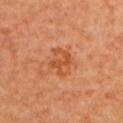Assessment: Part of a total-body skin-imaging series; this lesion was reviewed on a skin check and was not flagged for biopsy. Clinical summary: The subject is female. On the back. Cropped from a total-body skin-imaging series; the visible field is about 15 mm. The lesion's longest dimension is about 3.5 mm. This is a cross-polarized tile.